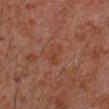<record>
  <lighting>cross-polarized</lighting>
  <site>right forearm</site>
  <lesion_size>
    <long_diameter_mm_approx>2.5</long_diameter_mm_approx>
  </lesion_size>
  <patient>
    <sex>male</sex>
    <age_approx>30</age_approx>
  </patient>
  <image>
    <source>total-body photography crop</source>
    <field_of_view_mm>15</field_of_view_mm>
  </image>
</record>